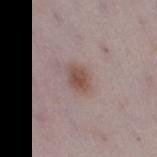Q: Was this lesion biopsied?
A: imaged on a skin check; not biopsied
Q: What lighting was used for the tile?
A: white-light
Q: Lesion location?
A: the right thigh
Q: What is the imaging modality?
A: ~15 mm crop, total-body skin-cancer survey
Q: What are the patient's age and sex?
A: female, aged approximately 30